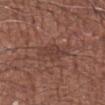notes: no biopsy performed (imaged during a skin exam)
illumination: white-light illumination
body site: the left forearm
lesion size: ~3 mm (longest diameter)
image source: total-body-photography crop, ~15 mm field of view
automated lesion analysis: a footprint of about 6 mm² and a shape-asymmetry score of about 0.3 (0 = symmetric)
patient: male, aged 68–72